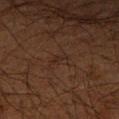Recorded during total-body skin imaging; not selected for excision or biopsy. A 15 mm close-up extracted from a 3D total-body photography capture. The tile uses cross-polarized illumination. The lesion is located on the right forearm. A male patient, about 65 years old. Measured at roughly 2.5 mm in maximum diameter. The total-body-photography lesion software estimated internal color variation of about 0 on a 0–10 scale and radial color variation of about 0. It also reported a detector confidence of about 95 out of 100 that the crop contains a lesion.